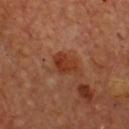illumination: cross-polarized
site: the chest
patient: male, roughly 50 years of age
image source: total-body-photography crop, ~15 mm field of view